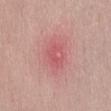Notes:
- notes — no biopsy performed (imaged during a skin exam)
- diameter — about 3 mm
- image — 15 mm crop, total-body photography
- body site — the abdomen
- subject — male, aged approximately 40
- lighting — white-light illumination
- automated metrics — a footprint of about 5.5 mm² and an eccentricity of roughly 0.7; a mean CIELAB color near L≈57 a*≈33 b*≈23, roughly 7 lightness units darker than nearby skin, and a lesion-to-skin contrast of about 4.5 (normalized; higher = more distinct); a border-irregularity rating of about 2/10; a classifier nevus-likeness of about 0/100 and a detector confidence of about 100 out of 100 that the crop contains a lesion